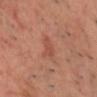Q: Was this lesion biopsied?
A: total-body-photography surveillance lesion; no biopsy
Q: What is the anatomic site?
A: the chest
Q: What kind of image is this?
A: total-body-photography crop, ~15 mm field of view
Q: What are the patient's age and sex?
A: male, roughly 50 years of age
Q: Illumination type?
A: cross-polarized